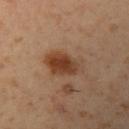Q: Is there a histopathology result?
A: no biopsy performed (imaged during a skin exam)
Q: Where on the body is the lesion?
A: the arm
Q: What kind of image is this?
A: ~15 mm crop, total-body skin-cancer survey
Q: What did automated image analysis measure?
A: a symmetry-axis asymmetry near 0.2; a mean CIELAB color near L≈40 a*≈20 b*≈30, roughly 11 lightness units darker than nearby skin, and a lesion-to-skin contrast of about 9.5 (normalized; higher = more distinct); a border-irregularity index near 2/10, a within-lesion color-variation index near 5.5/10, and radial color variation of about 1.5
Q: How was the tile lit?
A: cross-polarized illumination
Q: Patient demographics?
A: male, about 55 years old
Q: Lesion size?
A: ~5 mm (longest diameter)